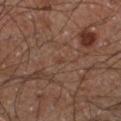Imaged during a routine full-body skin examination; the lesion was not biopsied and no histopathology is available. The lesion's longest dimension is about 1 mm. A male patient, aged 58 to 62. The lesion is located on the right thigh. The total-body-photography lesion software estimated an automated nevus-likeness rating near 0 out of 100 and a detector confidence of about 95 out of 100 that the crop contains a lesion. Cropped from a total-body skin-imaging series; the visible field is about 15 mm. The tile uses cross-polarized illumination.